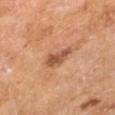Q: Was this lesion biopsied?
A: catalogued during a skin exam; not biopsied
Q: How was the tile lit?
A: cross-polarized illumination
Q: What kind of image is this?
A: ~15 mm tile from a whole-body skin photo
Q: Where on the body is the lesion?
A: the right lower leg
Q: What did automated image analysis measure?
A: a footprint of about 5.5 mm² and an eccentricity of roughly 0.75; a border-irregularity rating of about 3.5/10, a color-variation rating of about 2.5/10, and radial color variation of about 1
Q: What are the patient's age and sex?
A: male, roughly 65 years of age